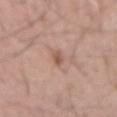No biopsy was performed on this lesion — it was imaged during a full skin examination and was not determined to be concerning. A male patient in their mid- to late 60s. Cropped from a whole-body photographic skin survey; the tile spans about 15 mm. Measured at roughly 2.5 mm in maximum diameter. The lesion is located on the mid back.